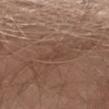Imaged during a routine full-body skin examination; the lesion was not biopsied and no histopathology is available.
Located on the left thigh.
Cropped from a whole-body photographic skin survey; the tile spans about 15 mm.
A male subject, roughly 30 years of age.